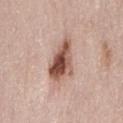No biopsy was performed on this lesion — it was imaged during a full skin examination and was not determined to be concerning. A female subject aged around 50. A close-up tile cropped from a whole-body skin photograph, about 15 mm across. The recorded lesion diameter is about 5 mm. The tile uses white-light illumination. On the back.